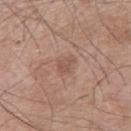Clinical impression: Part of a total-body skin-imaging series; this lesion was reviewed on a skin check and was not flagged for biopsy.